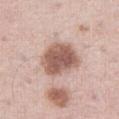Imaged during a routine full-body skin examination; the lesion was not biopsied and no histopathology is available. The patient is a male roughly 75 years of age. On the right thigh. Cropped from a total-body skin-imaging series; the visible field is about 15 mm. This is a white-light tile. The lesion's longest dimension is about 5 mm.